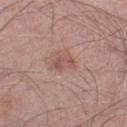Findings:
* notes: no biopsy performed (imaged during a skin exam)
* acquisition: total-body-photography crop, ~15 mm field of view
* subject: male, about 70 years old
* TBP lesion metrics: a classifier nevus-likeness of about 20/100 and lesion-presence confidence of about 100/100
* tile lighting: white-light
* location: the right thigh
* size: ≈2.5 mm